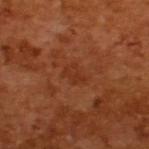Captured under cross-polarized illumination. Measured at roughly 3 mm in maximum diameter. A roughly 15 mm field-of-view crop from a total-body skin photograph. A male patient, approximately 65 years of age.